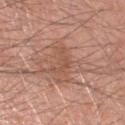{
  "biopsy_status": "not biopsied; imaged during a skin examination",
  "lesion_size": {
    "long_diameter_mm_approx": 3.5
  },
  "lighting": "white-light",
  "image": {
    "source": "total-body photography crop",
    "field_of_view_mm": 15
  },
  "site": "head or neck",
  "automated_metrics": {
    "cielab_L": 52,
    "cielab_a": 21,
    "cielab_b": 29,
    "vs_skin_darker_L": 7.0,
    "vs_skin_contrast_norm": 5.0,
    "border_irregularity_0_10": 8.0,
    "color_variation_0_10": 1.0
  },
  "patient": {
    "sex": "male",
    "age_approx": 50
  }
}